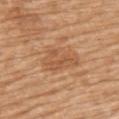No biopsy was performed on this lesion — it was imaged during a full skin examination and was not determined to be concerning.
A 15 mm close-up tile from a total-body photography series done for melanoma screening.
Imaged with white-light lighting.
A male subject aged around 70.
Longest diameter approximately 4.5 mm.
The lesion is on the upper back.